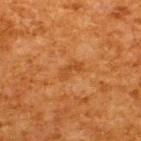Clinical impression:
No biopsy was performed on this lesion — it was imaged during a full skin examination and was not determined to be concerning.
Clinical summary:
A region of skin cropped from a whole-body photographic capture, roughly 15 mm wide. The patient is a male aged approximately 65. From the upper back. This is a cross-polarized tile. The lesion-visualizer software estimated a lesion color around L≈40 a*≈24 b*≈38 in CIELAB and a lesion–skin lightness drop of about 6. The analysis additionally found a border-irregularity index near 4/10, a within-lesion color-variation index near 1.5/10, and peripheral color asymmetry of about 0.5. About 3 mm across.